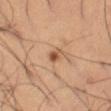Findings:
* workup — total-body-photography surveillance lesion; no biopsy
* image — ~15 mm crop, total-body skin-cancer survey
* location — the left thigh
* illumination — cross-polarized illumination
* subject — male, aged approximately 65